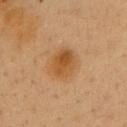– notes · catalogued during a skin exam; not biopsied
– image · 15 mm crop, total-body photography
– diameter · ~4 mm (longest diameter)
– image-analysis metrics · a lesion color around L≈44 a*≈19 b*≈35 in CIELAB, about 8 CIELAB-L* units darker than the surrounding skin, and a normalized lesion–skin contrast near 7.5; a nevus-likeness score of about 95/100 and lesion-presence confidence of about 100/100
– anatomic site · the upper back
– illumination · cross-polarized
– patient · female, aged 38–42